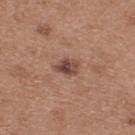Impression: Imaged during a routine full-body skin examination; the lesion was not biopsied and no histopathology is available. Image and clinical context: An algorithmic analysis of the crop reported a footprint of about 4.5 mm² and a symmetry-axis asymmetry near 0.25. It also reported a lesion color around L≈45 a*≈21 b*≈26 in CIELAB and a lesion–skin lightness drop of about 12. The analysis additionally found a border-irregularity index near 2.5/10, internal color variation of about 5 on a 0–10 scale, and radial color variation of about 2. The software also gave a nevus-likeness score of about 50/100 and a lesion-detection confidence of about 100/100. Located on the upper back. The tile uses white-light illumination. Cropped from a whole-body photographic skin survey; the tile spans about 15 mm. A female patient aged around 40.